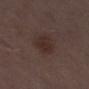The lesion was tiled from a total-body skin photograph and was not biopsied.
From the left thigh.
About 3 mm across.
Captured under white-light illumination.
A male patient aged 68 to 72.
The total-body-photography lesion software estimated a classifier nevus-likeness of about 50/100 and a lesion-detection confidence of about 100/100.
A 15 mm crop from a total-body photograph taken for skin-cancer surveillance.